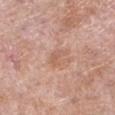The lesion was tiled from a total-body skin photograph and was not biopsied.